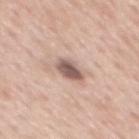Impression: Imaged during a routine full-body skin examination; the lesion was not biopsied and no histopathology is available. Acquisition and patient details: An algorithmic analysis of the crop reported an outline eccentricity of about 0.75 (0 = round, 1 = elongated) and a shape-asymmetry score of about 0.25 (0 = symmetric). The software also gave a lesion color around L≈57 a*≈17 b*≈22 in CIELAB and a lesion-to-skin contrast of about 10 (normalized; higher = more distinct). The analysis additionally found a nevus-likeness score of about 65/100. Cropped from a whole-body photographic skin survey; the tile spans about 15 mm. A male patient aged 58 to 62. About 3.5 mm across. The tile uses white-light illumination. The lesion is on the mid back.